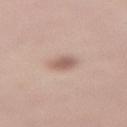Clinical impression:
Imaged during a routine full-body skin examination; the lesion was not biopsied and no histopathology is available.
Context:
A 15 mm close-up tile from a total-body photography series done for melanoma screening. On the lower back. A female patient aged 28–32.